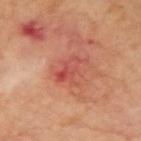Q: Was a biopsy performed?
A: total-body-photography surveillance lesion; no biopsy
Q: What is the lesion's diameter?
A: ≈3.5 mm
Q: Where on the body is the lesion?
A: the right upper arm
Q: What did automated image analysis measure?
A: an area of roughly 9.5 mm², an eccentricity of roughly 0.6, and two-axis asymmetry of about 0.4; a mean CIELAB color near L≈51 a*≈32 b*≈30, about 7 CIELAB-L* units darker than the surrounding skin, and a lesion-to-skin contrast of about 5.5 (normalized; higher = more distinct); a nevus-likeness score of about 0/100 and lesion-presence confidence of about 100/100
Q: What is the imaging modality?
A: ~15 mm crop, total-body skin-cancer survey
Q: How was the tile lit?
A: cross-polarized
Q: Patient demographics?
A: male, about 70 years old